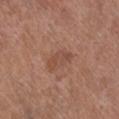Q: What did automated image analysis measure?
A: a footprint of about 5 mm², a shape eccentricity near 0.9, and a shape-asymmetry score of about 0.25 (0 = symmetric); an average lesion color of about L≈48 a*≈22 b*≈28 (CIELAB), roughly 7 lightness units darker than nearby skin, and a lesion-to-skin contrast of about 5.5 (normalized; higher = more distinct); border irregularity of about 3 on a 0–10 scale, a color-variation rating of about 3.5/10, and radial color variation of about 1; an automated nevus-likeness rating near 0 out of 100 and lesion-presence confidence of about 100/100
Q: Patient demographics?
A: female, aged 63–67
Q: What is the anatomic site?
A: the left leg
Q: How large is the lesion?
A: ≈3.5 mm
Q: What is the imaging modality?
A: total-body-photography crop, ~15 mm field of view